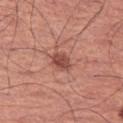<case>
<lesion_size>
  <long_diameter_mm_approx>2.5</long_diameter_mm_approx>
</lesion_size>
<patient>
  <sex>male</sex>
  <age_approx>65</age_approx>
</patient>
<automated_metrics>
  <area_mm2_approx>4.5</area_mm2_approx>
  <shape_asymmetry>0.15</shape_asymmetry>
  <cielab_L>48</cielab_L>
  <cielab_a>27</cielab_a>
  <cielab_b>27</cielab_b>
  <vs_skin_darker_L>12.0</vs_skin_darker_L>
  <vs_skin_contrast_norm>8.5</vs_skin_contrast_norm>
  <border_irregularity_0_10>1.5</border_irregularity_0_10>
  <color_variation_0_10>2.0</color_variation_0_10>
  <peripheral_color_asymmetry>1.0</peripheral_color_asymmetry>
  <nevus_likeness_0_100>70</nevus_likeness_0_100>
  <lesion_detection_confidence_0_100>100</lesion_detection_confidence_0_100>
</automated_metrics>
<lighting>white-light</lighting>
<site>left thigh</site>
<image>
  <source>total-body photography crop</source>
  <field_of_view_mm>15</field_of_view_mm>
</image>
</case>